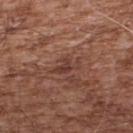  lighting: white-light
  site: upper back
  patient:
    sex: male
    age_approx: 55
  lesion_size:
    long_diameter_mm_approx: 3.5
  image:
    source: total-body photography crop
    field_of_view_mm: 15